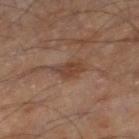Imaged during a routine full-body skin examination; the lesion was not biopsied and no histopathology is available. A male patient, in their mid-50s. Captured under cross-polarized illumination. A 15 mm close-up extracted from a 3D total-body photography capture. From the leg. The recorded lesion diameter is about 3.5 mm.